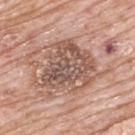Recorded during total-body skin imaging; not selected for excision or biopsy. A male subject aged around 80. A lesion tile, about 15 mm wide, cut from a 3D total-body photograph. About 7 mm across. The lesion is on the back. Automated image analysis of the tile measured an outline eccentricity of about 0.5 (0 = round, 1 = elongated) and a shape-asymmetry score of about 0.35 (0 = symmetric). And it measured a mean CIELAB color near L≈55 a*≈19 b*≈25, a lesion–skin lightness drop of about 11, and a normalized lesion–skin contrast near 7.5. It also reported a nevus-likeness score of about 0/100.